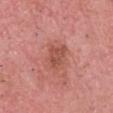Clinical impression: The lesion was tiled from a total-body skin photograph and was not biopsied. Clinical summary: The patient is a male aged 53–57. On the head or neck. A 15 mm close-up tile from a total-body photography series done for melanoma screening.